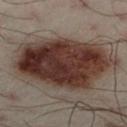notes: no biopsy performed (imaged during a skin exam) | site: the left lower leg | imaging modality: total-body-photography crop, ~15 mm field of view | patient: male, aged 48–52.